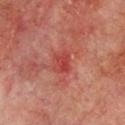Clinical summary: A male patient, aged 63 to 67. Imaged with cross-polarized lighting. The lesion is located on the upper back. A 15 mm close-up extracted from a 3D total-body photography capture.